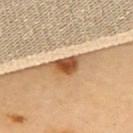Imaged during a routine full-body skin examination; the lesion was not biopsied and no histopathology is available.
A lesion tile, about 15 mm wide, cut from a 3D total-body photograph.
This is a cross-polarized tile.
A female patient, aged 58 to 62.
The lesion is on the upper back.
Automated image analysis of the tile measured a border-irregularity index near 3.5/10, internal color variation of about 6.5 on a 0–10 scale, and radial color variation of about 1.5. And it measured an automated nevus-likeness rating near 100 out of 100.